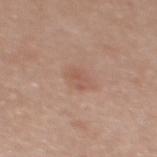Q: Was this lesion biopsied?
A: imaged on a skin check; not biopsied
Q: What kind of image is this?
A: ~15 mm crop, total-body skin-cancer survey
Q: Where on the body is the lesion?
A: the upper back
Q: What is the lesion's diameter?
A: ≈3 mm
Q: Who is the patient?
A: female, in their mid- to late 30s
Q: What lighting was used for the tile?
A: white-light illumination